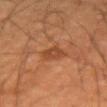| feature | finding |
|---|---|
| follow-up | no biopsy performed (imaged during a skin exam) |
| size | ≈3 mm |
| patient | male, aged approximately 55 |
| illumination | cross-polarized illumination |
| imaging modality | ~15 mm crop, total-body skin-cancer survey |
| body site | the arm |